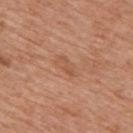The lesion was photographed on a routine skin check and not biopsied; there is no pathology result. Located on the upper back. Longest diameter approximately 3.5 mm. A male subject aged 68–72. Imaged with white-light lighting. Cropped from a total-body skin-imaging series; the visible field is about 15 mm.